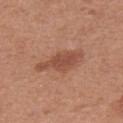Impression:
The lesion was photographed on a routine skin check and not biopsied; there is no pathology result.
Acquisition and patient details:
The tile uses white-light illumination. Approximately 6 mm at its widest. The lesion is on the left thigh. A female subject aged approximately 40. Cropped from a whole-body photographic skin survey; the tile spans about 15 mm. The total-body-photography lesion software estimated a lesion color around L≈50 a*≈24 b*≈30 in CIELAB and roughly 10 lightness units darker than nearby skin. And it measured border irregularity of about 4 on a 0–10 scale, internal color variation of about 2 on a 0–10 scale, and a peripheral color-asymmetry measure near 0.5. The software also gave a classifier nevus-likeness of about 50/100.